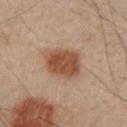Clinical impression:
The lesion was tiled from a total-body skin photograph and was not biopsied.
Image and clinical context:
On the left upper arm. A male subject about 50 years old. A lesion tile, about 15 mm wide, cut from a 3D total-body photograph.